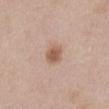* follow-up · no biopsy performed (imaged during a skin exam)
* subject · female, aged 38 to 42
* automated lesion analysis · a footprint of about 5.5 mm² and an outline eccentricity of about 0.45 (0 = round, 1 = elongated); roughly 11 lightness units darker than nearby skin and a normalized border contrast of about 7.5
* illumination · white-light illumination
* body site · the abdomen
* imaging modality · 15 mm crop, total-body photography